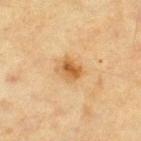Image and clinical context: A 15 mm close-up tile from a total-body photography series done for melanoma screening. On the leg. A female patient about 55 years old. Automated image analysis of the tile measured a footprint of about 5 mm² and an outline eccentricity of about 0.45 (0 = round, 1 = elongated). The analysis additionally found border irregularity of about 2 on a 0–10 scale and radial color variation of about 1.5. The software also gave lesion-presence confidence of about 100/100. Imaged with cross-polarized lighting. Longest diameter approximately 2.5 mm.